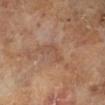Notes:
• biopsy status — total-body-photography surveillance lesion; no biopsy
• subject — male, aged 63 to 67
• image — ~15 mm crop, total-body skin-cancer survey